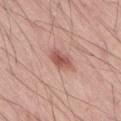Impression:
The lesion was photographed on a routine skin check and not biopsied; there is no pathology result.
Context:
From the right thigh. The patient is a male aged around 55. Automated image analysis of the tile measured roughly 12 lightness units darker than nearby skin and a lesion-to-skin contrast of about 8 (normalized; higher = more distinct). The analysis additionally found a border-irregularity index near 2/10 and a within-lesion color-variation index near 3/10. Approximately 2.5 mm at its widest. A lesion tile, about 15 mm wide, cut from a 3D total-body photograph. The tile uses white-light illumination.